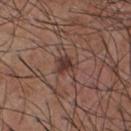Recorded during total-body skin imaging; not selected for excision or biopsy. The tile uses white-light illumination. A male subject, aged 53–57. The lesion's longest dimension is about 2.5 mm. The lesion-visualizer software estimated a lesion color around L≈37 a*≈19 b*≈21 in CIELAB, roughly 9 lightness units darker than nearby skin, and a lesion-to-skin contrast of about 8.5 (normalized; higher = more distinct). A close-up tile cropped from a whole-body skin photograph, about 15 mm across. On the chest.